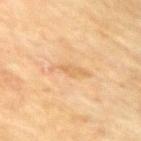size: about 4.5 mm | location: the arm | patient: female, in their 80s | acquisition: ~15 mm tile from a whole-body skin photo | lighting: cross-polarized.